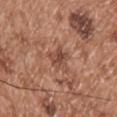Notes:
- follow-up — imaged on a skin check; not biopsied
- lesion diameter — ≈3 mm
- anatomic site — the chest
- image source — ~15 mm tile from a whole-body skin photo
- subject — male, approximately 55 years of age
- TBP lesion metrics — a mean CIELAB color near L≈47 a*≈22 b*≈28 and a normalized border contrast of about 7; border irregularity of about 3 on a 0–10 scale, a within-lesion color-variation index near 4/10, and a peripheral color-asymmetry measure near 1.5; a classifier nevus-likeness of about 0/100 and a lesion-detection confidence of about 100/100